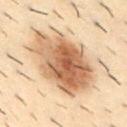Q: Was this lesion biopsied?
A: no biopsy performed (imaged during a skin exam)
Q: What is the lesion's diameter?
A: ~9 mm (longest diameter)
Q: How was the tile lit?
A: cross-polarized
Q: Lesion location?
A: the abdomen
Q: What are the patient's age and sex?
A: male, aged 28–32
Q: What is the imaging modality?
A: total-body-photography crop, ~15 mm field of view